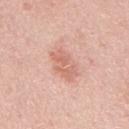– notes: imaged on a skin check; not biopsied
– size: ~4 mm (longest diameter)
– patient: male, in their mid- to late 40s
– anatomic site: the mid back
– image source: ~15 mm tile from a whole-body skin photo
– automated lesion analysis: an area of roughly 8 mm²; border irregularity of about 2.5 on a 0–10 scale, internal color variation of about 3 on a 0–10 scale, and peripheral color asymmetry of about 1
– lighting: white-light